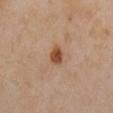Part of a total-body skin-imaging series; this lesion was reviewed on a skin check and was not flagged for biopsy.
A female subject aged 38–42.
The lesion is located on the chest.
A 15 mm close-up tile from a total-body photography series done for melanoma screening.